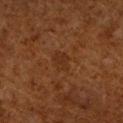The lesion was tiled from a total-body skin photograph and was not biopsied.
Longest diameter approximately 2.5 mm.
A 15 mm close-up extracted from a 3D total-body photography capture.
This is a cross-polarized tile.
On the arm.
The subject is a male about 60 years old.
The total-body-photography lesion software estimated a lesion area of about 4.5 mm² and a shape-asymmetry score of about 0.2 (0 = symmetric).